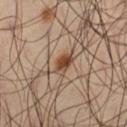Assessment: Captured during whole-body skin photography for melanoma surveillance; the lesion was not biopsied. Background: The patient is a male in their 60s. The lesion is located on the left thigh. Automated image analysis of the tile measured a lesion–skin lightness drop of about 12 and a lesion-to-skin contrast of about 10 (normalized; higher = more distinct). A region of skin cropped from a whole-body photographic capture, roughly 15 mm wide.